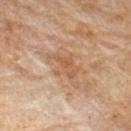Recorded during total-body skin imaging; not selected for excision or biopsy.
Cropped from a whole-body photographic skin survey; the tile spans about 15 mm.
The tile uses cross-polarized illumination.
The lesion's longest dimension is about 5 mm.
On the upper back.
A male patient, in their mid- to late 80s or older.